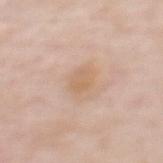Q: Was this lesion biopsied?
A: total-body-photography surveillance lesion; no biopsy
Q: Lesion location?
A: the upper back
Q: Who is the patient?
A: female, about 50 years old
Q: Automated lesion metrics?
A: an area of roughly 4.5 mm², an outline eccentricity of about 0.5 (0 = round, 1 = elongated), and a symmetry-axis asymmetry near 0.15; a mean CIELAB color near L≈64 a*≈17 b*≈33, roughly 6 lightness units darker than nearby skin, and a normalized lesion–skin contrast near 5.5; a within-lesion color-variation index near 2.5/10 and radial color variation of about 1
Q: What is the lesion's diameter?
A: ~2.5 mm (longest diameter)
Q: What kind of image is this?
A: ~15 mm tile from a whole-body skin photo
Q: What lighting was used for the tile?
A: white-light illumination